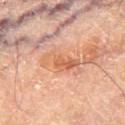biopsy status: total-body-photography surveillance lesion; no biopsy
patient: male, aged approximately 70
acquisition: 15 mm crop, total-body photography
anatomic site: the right thigh